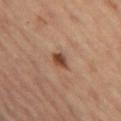Q: Was a biopsy performed?
A: catalogued during a skin exam; not biopsied
Q: What is the imaging modality?
A: ~15 mm crop, total-body skin-cancer survey
Q: Automated lesion metrics?
A: two-axis asymmetry of about 0.25; a border-irregularity rating of about 2.5/10; a detector confidence of about 100 out of 100 that the crop contains a lesion
Q: Who is the patient?
A: female, aged 53–57
Q: How large is the lesion?
A: about 2.5 mm
Q: What is the anatomic site?
A: the left thigh
Q: What lighting was used for the tile?
A: cross-polarized illumination